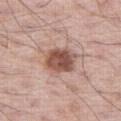Q: Was this lesion biopsied?
A: total-body-photography surveillance lesion; no biopsy
Q: Automated lesion metrics?
A: border irregularity of about 1.5 on a 0–10 scale and a within-lesion color-variation index near 5/10; a nevus-likeness score of about 95/100 and a lesion-detection confidence of about 100/100
Q: How was this image acquired?
A: ~15 mm crop, total-body skin-cancer survey
Q: Lesion size?
A: ~4 mm (longest diameter)
Q: Lesion location?
A: the right thigh
Q: Who is the patient?
A: male, in their 60s
Q: How was the tile lit?
A: white-light